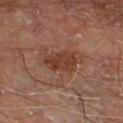Assessment:
The lesion was photographed on a routine skin check and not biopsied; there is no pathology result.
Clinical summary:
Located on the left lower leg. A region of skin cropped from a whole-body photographic capture, roughly 15 mm wide. The subject is a male approximately 70 years of age.